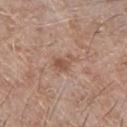The lesion was tiled from a total-body skin photograph and was not biopsied.
This is a white-light tile.
The subject is a male aged 63–67.
The lesion's longest dimension is about 3.5 mm.
Located on the chest.
A 15 mm crop from a total-body photograph taken for skin-cancer surveillance.